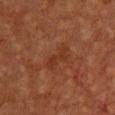Clinical summary:
This is a cross-polarized tile. An algorithmic analysis of the crop reported a lesion–skin lightness drop of about 5 and a lesion-to-skin contrast of about 5.5 (normalized; higher = more distinct). And it measured a border-irregularity index near 6.5/10, a color-variation rating of about 0.5/10, and a peripheral color-asymmetry measure near 0. Located on the chest. A region of skin cropped from a whole-body photographic capture, roughly 15 mm wide. Approximately 4 mm at its widest. A female patient approximately 40 years of age.